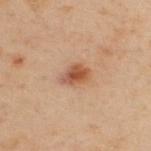size: ~3 mm (longest diameter) | image-analysis metrics: an area of roughly 5.5 mm² and an outline eccentricity of about 0.7 (0 = round, 1 = elongated); border irregularity of about 2.5 on a 0–10 scale, a color-variation rating of about 5.5/10, and a peripheral color-asymmetry measure near 2; an automated nevus-likeness rating near 95 out of 100 and lesion-presence confidence of about 100/100 | acquisition: total-body-photography crop, ~15 mm field of view | anatomic site: the upper back | subject: male, approximately 50 years of age.